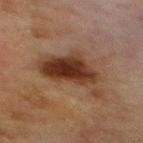subject: male, aged approximately 70
location: the mid back
automated lesion analysis: a lesion area of about 20 mm², a shape eccentricity near 0.75, and two-axis asymmetry of about 0.4; an average lesion color of about L≈29 a*≈17 b*≈25 (CIELAB), roughly 11 lightness units darker than nearby skin, and a normalized lesion–skin contrast near 11; an automated nevus-likeness rating near 100 out of 100
illumination: cross-polarized illumination
lesion size: ≈7 mm
image source: ~15 mm tile from a whole-body skin photo